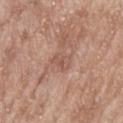{"biopsy_status": "not biopsied; imaged during a skin examination", "image": {"source": "total-body photography crop", "field_of_view_mm": 15}, "lesion_size": {"long_diameter_mm_approx": 2.5}, "automated_metrics": {"border_irregularity_0_10": 3.0, "color_variation_0_10": 2.5, "peripheral_color_asymmetry": 1.0, "nevus_likeness_0_100": 0, "lesion_detection_confidence_0_100": 100}, "site": "upper back", "lighting": "white-light", "patient": {"sex": "male", "age_approx": 65}}